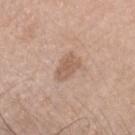Q: Was a biopsy performed?
A: total-body-photography surveillance lesion; no biopsy
Q: What is the anatomic site?
A: the head or neck
Q: How large is the lesion?
A: ≈3.5 mm
Q: How was this image acquired?
A: 15 mm crop, total-body photography
Q: Who is the patient?
A: female, aged 38 to 42
Q: How was the tile lit?
A: white-light illumination